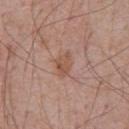Assessment: This lesion was catalogued during total-body skin photography and was not selected for biopsy. Clinical summary: Imaged with white-light lighting. The subject is a male aged 68–72. The lesion's longest dimension is about 3 mm. Automated image analysis of the tile measured a lesion area of about 4.5 mm², an eccentricity of roughly 0.8, and a shape-asymmetry score of about 0.3 (0 = symmetric). The software also gave a mean CIELAB color near L≈53 a*≈21 b*≈29 and a normalized border contrast of about 6.5. On the chest. A 15 mm crop from a total-body photograph taken for skin-cancer surveillance.